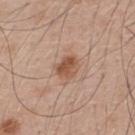Assessment: The lesion was tiled from a total-body skin photograph and was not biopsied. Acquisition and patient details: The recorded lesion diameter is about 3 mm. The lesion is located on the upper back. A 15 mm crop from a total-body photograph taken for skin-cancer surveillance. A male patient, approximately 50 years of age. Captured under white-light illumination.